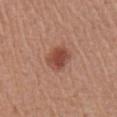Q: Is there a histopathology result?
A: imaged on a skin check; not biopsied
Q: What is the imaging modality?
A: 15 mm crop, total-body photography
Q: What lighting was used for the tile?
A: white-light
Q: Who is the patient?
A: male, in their mid-60s
Q: Lesion size?
A: ≈3.5 mm
Q: What did automated image analysis measure?
A: a mean CIELAB color near L≈47 a*≈25 b*≈29, about 11 CIELAB-L* units darker than the surrounding skin, and a normalized lesion–skin contrast near 8.5; a within-lesion color-variation index near 3/10 and peripheral color asymmetry of about 1
Q: What is the anatomic site?
A: the chest